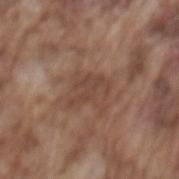biopsy status: catalogued during a skin exam; not biopsied | lesion diameter: about 4 mm | acquisition: total-body-photography crop, ~15 mm field of view | patient: male, about 75 years old | anatomic site: the back | illumination: white-light | image-analysis metrics: an area of roughly 6 mm², an outline eccentricity of about 0.8 (0 = round, 1 = elongated), and a shape-asymmetry score of about 0.55 (0 = symmetric); a mean CIELAB color near L≈43 a*≈18 b*≈26, roughly 6 lightness units darker than nearby skin, and a normalized lesion–skin contrast near 5.5; border irregularity of about 9 on a 0–10 scale and a within-lesion color-variation index near 0.5/10.